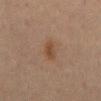Q: Was this lesion biopsied?
A: total-body-photography surveillance lesion; no biopsy
Q: Lesion size?
A: about 3 mm
Q: Where on the body is the lesion?
A: the mid back
Q: What is the imaging modality?
A: ~15 mm crop, total-body skin-cancer survey
Q: What are the patient's age and sex?
A: male, aged 68–72
Q: Automated lesion metrics?
A: an area of roughly 4 mm², an outline eccentricity of about 0.9 (0 = round, 1 = elongated), and a symmetry-axis asymmetry near 0.25; a lesion color around L≈37 a*≈16 b*≈26 in CIELAB, roughly 6 lightness units darker than nearby skin, and a normalized border contrast of about 6.5; peripheral color asymmetry of about 1; a detector confidence of about 100 out of 100 that the crop contains a lesion